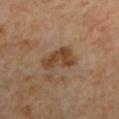Clinical impression:
This lesion was catalogued during total-body skin photography and was not selected for biopsy.
Context:
Captured under cross-polarized illumination. A female subject, aged 48–52. From the left lower leg. A roughly 15 mm field-of-view crop from a total-body skin photograph. Measured at roughly 4 mm in maximum diameter. Automated image analysis of the tile measured an average lesion color of about L≈45 a*≈19 b*≈33 (CIELAB), a lesion–skin lightness drop of about 10, and a normalized lesion–skin contrast near 8.5. The software also gave a classifier nevus-likeness of about 5/100 and a lesion-detection confidence of about 100/100.